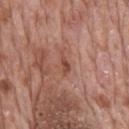Q: Is there a histopathology result?
A: catalogued during a skin exam; not biopsied
Q: Illumination type?
A: white-light illumination
Q: What is the lesion's diameter?
A: about 3 mm
Q: How was this image acquired?
A: total-body-photography crop, ~15 mm field of view
Q: Who is the patient?
A: male, aged around 70
Q: Lesion location?
A: the mid back